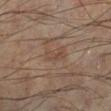Impression:
The lesion was photographed on a routine skin check and not biopsied; there is no pathology result.
Context:
Imaged with cross-polarized lighting. Longest diameter approximately 3 mm. The lesion is located on the left lower leg. A male patient aged approximately 55. A 15 mm close-up extracted from a 3D total-body photography capture.